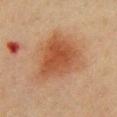About 6.5 mm across.
On the chest.
A 15 mm crop from a total-body photograph taken for skin-cancer surveillance.
Imaged with cross-polarized lighting.
A female subject aged around 40.